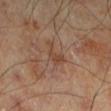follow-up: imaged on a skin check; not biopsied | body site: the left lower leg | patient: male, aged around 70 | automated lesion analysis: a footprint of about 4 mm², an outline eccentricity of about 0.75 (0 = round, 1 = elongated), and a symmetry-axis asymmetry near 0.55; about 7 CIELAB-L* units darker than the surrounding skin and a normalized lesion–skin contrast near 6 | lighting: cross-polarized | size: ~3 mm (longest diameter) | image: ~15 mm tile from a whole-body skin photo.